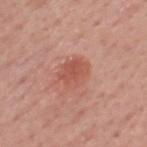Imaged during a routine full-body skin examination; the lesion was not biopsied and no histopathology is available. A male patient aged approximately 50. Approximately 4 mm at its widest. The tile uses white-light illumination. A 15 mm close-up tile from a total-body photography series done for melanoma screening. From the head or neck.